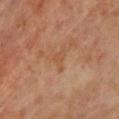This lesion was catalogued during total-body skin photography and was not selected for biopsy.
A female patient, roughly 65 years of age.
Captured under cross-polarized illumination.
Measured at roughly 3 mm in maximum diameter.
Located on the chest.
A 15 mm close-up extracted from a 3D total-body photography capture.